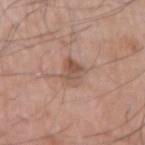notes: total-body-photography surveillance lesion; no biopsy
size: about 3.5 mm
subject: male, aged 73 to 77
location: the right upper arm
acquisition: 15 mm crop, total-body photography